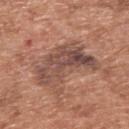follow-up=total-body-photography surveillance lesion; no biopsy | subject=male, in their mid-60s | acquisition=total-body-photography crop, ~15 mm field of view | location=the upper back | lesion diameter=≈7 mm | illumination=white-light.